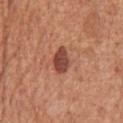Case summary:
* notes · total-body-photography surveillance lesion; no biopsy
* acquisition · 15 mm crop, total-body photography
* subject · male, roughly 65 years of age
* lighting · white-light
* lesion diameter · about 3.5 mm
* image-analysis metrics · internal color variation of about 3 on a 0–10 scale and radial color variation of about 1
* location · the front of the torso